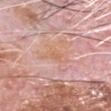The lesion was photographed on a routine skin check and not biopsied; there is no pathology result. A male patient, aged 78–82. Cropped from a whole-body photographic skin survey; the tile spans about 15 mm. Located on the head or neck. Longest diameter approximately 4 mm. The total-body-photography lesion software estimated a footprint of about 6 mm², a shape eccentricity near 0.75, and two-axis asymmetry of about 0.45. It also reported a lesion color around L≈65 a*≈21 b*≈31 in CIELAB, roughly 5 lightness units darker than nearby skin, and a lesion-to-skin contrast of about 6 (normalized; higher = more distinct). It also reported an automated nevus-likeness rating near 0 out of 100 and a lesion-detection confidence of about 100/100. Captured under white-light illumination.